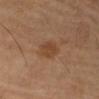Clinical impression:
Part of a total-body skin-imaging series; this lesion was reviewed on a skin check and was not flagged for biopsy.
Acquisition and patient details:
On the right upper arm. A male subject in their mid-60s. Measured at roughly 4 mm in maximum diameter. A close-up tile cropped from a whole-body skin photograph, about 15 mm across. The total-body-photography lesion software estimated a lesion color around L≈40 a*≈19 b*≈30 in CIELAB, about 7 CIELAB-L* units darker than the surrounding skin, and a normalized border contrast of about 6.5. The software also gave a border-irregularity index near 3/10, a within-lesion color-variation index near 2/10, and peripheral color asymmetry of about 1. It also reported a nevus-likeness score of about 60/100. The tile uses cross-polarized illumination.